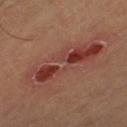Assessment: Recorded during total-body skin imaging; not selected for excision or biopsy. Image and clinical context: The subject is a male approximately 60 years of age. A roughly 15 mm field-of-view crop from a total-body skin photograph. Approximately 9 mm at its widest. This is a cross-polarized tile. From the left thigh. Automated tile analysis of the lesion measured a lesion–skin lightness drop of about 8 and a lesion-to-skin contrast of about 7.5 (normalized; higher = more distinct). And it measured a classifier nevus-likeness of about 0/100 and lesion-presence confidence of about 95/100.